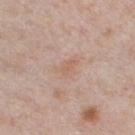Recorded during total-body skin imaging; not selected for excision or biopsy. The total-body-photography lesion software estimated internal color variation of about 1.5 on a 0–10 scale and peripheral color asymmetry of about 0.5. A 15 mm close-up tile from a total-body photography series done for melanoma screening. The patient is a male aged around 40. The lesion is located on the chest. Approximately 3 mm at its widest.